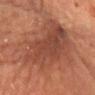Assessment:
No biopsy was performed on this lesion — it was imaged during a full skin examination and was not determined to be concerning.
Clinical summary:
Located on the chest. The subject is a female roughly 70 years of age. This image is a 15 mm lesion crop taken from a total-body photograph. The total-body-photography lesion software estimated a footprint of about 50 mm², an eccentricity of roughly 0.75, and two-axis asymmetry of about 0.3. And it measured a mean CIELAB color near L≈38 a*≈21 b*≈26, roughly 9 lightness units darker than nearby skin, and a lesion-to-skin contrast of about 7.5 (normalized; higher = more distinct).